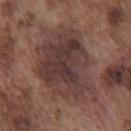Notes:
- biopsy status — total-body-photography surveillance lesion; no biopsy
- image source — 15 mm crop, total-body photography
- patient — male, in their mid- to late 70s
- site — the chest
- lesion diameter — ~8.5 mm (longest diameter)
- lighting — white-light
- automated lesion analysis — a border-irregularity index near 4.5/10 and internal color variation of about 5.5 on a 0–10 scale; an automated nevus-likeness rating near 15 out of 100 and a detector confidence of about 95 out of 100 that the crop contains a lesion